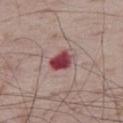<lesion>
  <biopsy_status>not biopsied; imaged during a skin examination</biopsy_status>
  <lesion_size>
    <long_diameter_mm_approx>3.5</long_diameter_mm_approx>
  </lesion_size>
  <automated_metrics>
    <area_mm2_approx>6.0</area_mm2_approx>
    <eccentricity>0.7</eccentricity>
    <shape_asymmetry>0.25</shape_asymmetry>
    <cielab_L>44</cielab_L>
    <cielab_a>30</cielab_a>
    <cielab_b>19</cielab_b>
    <vs_skin_darker_L>17.0</vs_skin_darker_L>
    <vs_skin_contrast_norm>12.5</vs_skin_contrast_norm>
  </automated_metrics>
  <lighting>white-light</lighting>
  <patient>
    <sex>male</sex>
    <age_approx>75</age_approx>
  </patient>
  <site>right thigh</site>
  <image>
    <source>total-body photography crop</source>
    <field_of_view_mm>15</field_of_view_mm>
  </image>
</lesion>